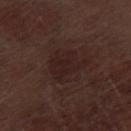This lesion was catalogued during total-body skin photography and was not selected for biopsy. The subject is a male roughly 70 years of age. On the left thigh. A lesion tile, about 15 mm wide, cut from a 3D total-body photograph.